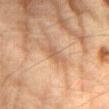notes: catalogued during a skin exam; not biopsied | lighting: cross-polarized illumination | imaging modality: 15 mm crop, total-body photography | site: the abdomen | patient: male, aged around 85 or older.